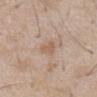Findings:
* workup — imaged on a skin check; not biopsied
* lighting — white-light illumination
* location — the abdomen
* diameter — ≈3 mm
* subject — male, approximately 60 years of age
* image source — total-body-photography crop, ~15 mm field of view